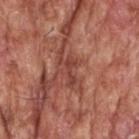Recorded during total-body skin imaging; not selected for excision or biopsy. The subject is a male aged around 60. This is a white-light tile. A lesion tile, about 15 mm wide, cut from a 3D total-body photograph. The lesion is located on the head or neck. Approximately 3.5 mm at its widest.